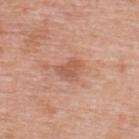This lesion was catalogued during total-body skin photography and was not selected for biopsy. On the upper back. Imaged with white-light lighting. The subject is a female aged 38–42. Automated tile analysis of the lesion measured a mean CIELAB color near L≈56 a*≈25 b*≈31. It also reported a border-irregularity rating of about 3/10, a color-variation rating of about 1.5/10, and a peripheral color-asymmetry measure near 0.5. A roughly 15 mm field-of-view crop from a total-body skin photograph.